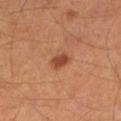Impression: The lesion was tiled from a total-body skin photograph and was not biopsied. Clinical summary: The lesion is located on the left lower leg. A male subject aged 63 to 67. Cropped from a total-body skin-imaging series; the visible field is about 15 mm.